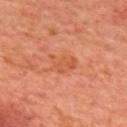<tbp_lesion>
<biopsy_status>not biopsied; imaged during a skin examination</biopsy_status>
<automated_metrics>
  <nevus_likeness_0_100>0</nevus_likeness_0_100>
  <lesion_detection_confidence_0_100>100</lesion_detection_confidence_0_100>
</automated_metrics>
<image>
  <source>total-body photography crop</source>
  <field_of_view_mm>15</field_of_view_mm>
</image>
<site>upper back</site>
<lighting>cross-polarized</lighting>
<lesion_size>
  <long_diameter_mm_approx>3.5</long_diameter_mm_approx>
</lesion_size>
<patient>
  <sex>male</sex>
  <age_approx>65</age_approx>
</patient>
</tbp_lesion>